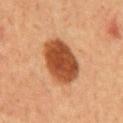biopsy status = catalogued during a skin exam; not biopsied
acquisition = 15 mm crop, total-body photography
site = the chest
diameter = about 6 mm
patient = male, aged around 60